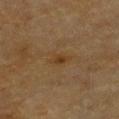workup — total-body-photography surveillance lesion; no biopsy
patient — male, aged approximately 85 or older
anatomic site — the chest
image — 15 mm crop, total-body photography